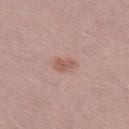workup — total-body-photography surveillance lesion; no biopsy
patient — male, aged 28–32
size — ≈2.5 mm
acquisition — 15 mm crop, total-body photography
automated lesion analysis — an area of roughly 3.5 mm², an eccentricity of roughly 0.85, and two-axis asymmetry of about 0.3
illumination — white-light